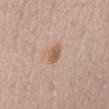image-analysis metrics = a color-variation rating of about 2.5/10 and radial color variation of about 1; tile lighting = white-light illumination; subject = female, about 65 years old; acquisition = total-body-photography crop, ~15 mm field of view; size = about 2.5 mm; location = the abdomen.